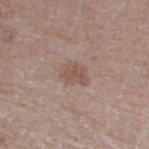Recorded during total-body skin imaging; not selected for excision or biopsy. The patient is a male aged 78–82. Located on the right lower leg. A 15 mm close-up extracted from a 3D total-body photography capture.